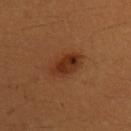Clinical impression:
Imaged during a routine full-body skin examination; the lesion was not biopsied and no histopathology is available.
Background:
Imaged with cross-polarized lighting. The patient is a female aged 38 to 42. The recorded lesion diameter is about 4.5 mm. A close-up tile cropped from a whole-body skin photograph, about 15 mm across. Located on the left upper arm. An algorithmic analysis of the crop reported a lesion area of about 9 mm², an outline eccentricity of about 0.85 (0 = round, 1 = elongated), and two-axis asymmetry of about 0.15. And it measured a nevus-likeness score of about 95/100 and lesion-presence confidence of about 100/100.